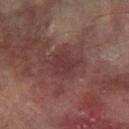Acquisition and patient details:
A 15 mm close-up tile from a total-body photography series done for melanoma screening. The lesion-visualizer software estimated a footprint of about 6 mm², a shape eccentricity near 0.6, and two-axis asymmetry of about 0.3. The software also gave a nevus-likeness score of about 0/100. On the arm. A male subject approximately 75 years of age.